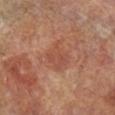Located on the left lower leg.
A 15 mm close-up tile from a total-body photography series done for melanoma screening.
The subject is a female in their mid- to late 70s.